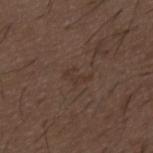workup: total-body-photography surveillance lesion; no biopsy | image: total-body-photography crop, ~15 mm field of view | site: the mid back | subject: male, aged around 50 | tile lighting: white-light illumination.